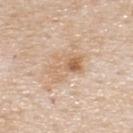The lesion was photographed on a routine skin check and not biopsied; there is no pathology result.
From the upper back.
A 15 mm crop from a total-body photograph taken for skin-cancer surveillance.
About 4.5 mm across.
The patient is a male aged 43 to 47.